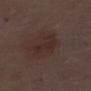Captured during whole-body skin photography for melanoma surveillance; the lesion was not biopsied. This image is a 15 mm lesion crop taken from a total-body photograph. The patient is a male roughly 70 years of age. On the left lower leg.